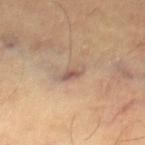{"lesion_size": {"long_diameter_mm_approx": 3.0}, "patient": {"sex": "male", "age_approx": 65}, "lighting": "cross-polarized", "site": "right lower leg", "automated_metrics": {"area_mm2_approx": 3.5, "eccentricity": 0.9, "shape_asymmetry": 0.25, "lesion_detection_confidence_0_100": 80}, "image": {"source": "total-body photography crop", "field_of_view_mm": 15}}